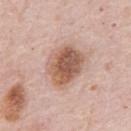Imaged during a routine full-body skin examination; the lesion was not biopsied and no histopathology is available.
Measured at roughly 5 mm in maximum diameter.
A 15 mm crop from a total-body photograph taken for skin-cancer surveillance.
Automated tile analysis of the lesion measured a lesion area of about 16 mm². The analysis additionally found a mean CIELAB color near L≈57 a*≈20 b*≈29, about 14 CIELAB-L* units darker than the surrounding skin, and a normalized border contrast of about 9.5. It also reported a nevus-likeness score of about 70/100 and a detector confidence of about 100 out of 100 that the crop contains a lesion.
This is a white-light tile.
The lesion is located on the chest.
A male subject, approximately 80 years of age.